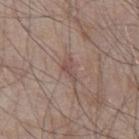Case summary:
- workup: total-body-photography surveillance lesion; no biopsy
- subject: male, aged around 65
- tile lighting: white-light
- automated metrics: an area of roughly 3 mm², an eccentricity of roughly 0.85, and a symmetry-axis asymmetry near 0.55
- image source: 15 mm crop, total-body photography
- lesion diameter: about 3 mm
- body site: the abdomen